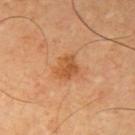Clinical impression:
Imaged during a routine full-body skin examination; the lesion was not biopsied and no histopathology is available.
Context:
This is a cross-polarized tile. A close-up tile cropped from a whole-body skin photograph, about 15 mm across. Located on the right upper arm. A male patient, aged 63 to 67. The recorded lesion diameter is about 3.5 mm. Automated image analysis of the tile measured a footprint of about 6.5 mm², an eccentricity of roughly 0.6, and two-axis asymmetry of about 0.3. The analysis additionally found a mean CIELAB color near L≈54 a*≈26 b*≈41 and a lesion–skin lightness drop of about 9. It also reported a border-irregularity index near 3/10, a color-variation rating of about 3/10, and radial color variation of about 1. The software also gave a classifier nevus-likeness of about 45/100 and a detector confidence of about 100 out of 100 that the crop contains a lesion.